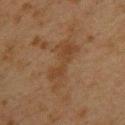The lesion was photographed on a routine skin check and not biopsied; there is no pathology result.
Located on the upper back.
A close-up tile cropped from a whole-body skin photograph, about 15 mm across.
A female patient, aged 38 to 42.